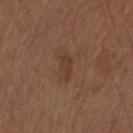Impression: Captured during whole-body skin photography for melanoma surveillance; the lesion was not biopsied. Clinical summary: This is a white-light tile. Cropped from a total-body skin-imaging series; the visible field is about 15 mm. Longest diameter approximately 3 mm. From the arm. Automated tile analysis of the lesion measured a lesion–skin lightness drop of about 6 and a lesion-to-skin contrast of about 5.5 (normalized; higher = more distinct). It also reported a border-irregularity rating of about 3/10 and a peripheral color-asymmetry measure near 0. The subject is a male about 75 years old.